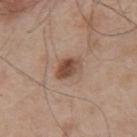| field | value |
|---|---|
| notes | catalogued during a skin exam; not biopsied |
| subject | male, in their mid- to late 50s |
| diameter | about 3 mm |
| imaging modality | 15 mm crop, total-body photography |
| illumination | white-light |
| body site | the upper back |